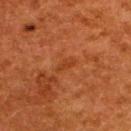follow-up=no biopsy performed (imaged during a skin exam).